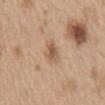  biopsy_status: not biopsied; imaged during a skin examination
  image:
    source: total-body photography crop
    field_of_view_mm: 15
  site: mid back
  patient:
    sex: male
    age_approx: 70